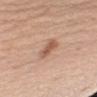This is a white-light tile. Cropped from a total-body skin-imaging series; the visible field is about 15 mm. A male subject aged around 60. The lesion-visualizer software estimated a lesion area of about 5 mm², a shape eccentricity near 0.8, and a shape-asymmetry score of about 0.25 (0 = symmetric). The lesion is located on the left upper arm.